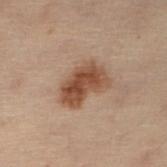Background:
The lesion is on the leg. The patient is a female aged around 50. This image is a 15 mm lesion crop taken from a total-body photograph. Captured under cross-polarized illumination.